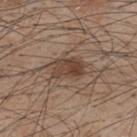Part of a total-body skin-imaging series; this lesion was reviewed on a skin check and was not flagged for biopsy.
The patient is a male approximately 45 years of age.
Located on the upper back.
Captured under white-light illumination.
Longest diameter approximately 4.5 mm.
A lesion tile, about 15 mm wide, cut from a 3D total-body photograph.